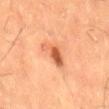Impression:
The lesion was photographed on a routine skin check and not biopsied; there is no pathology result.
Acquisition and patient details:
An algorithmic analysis of the crop reported a mean CIELAB color near L≈54 a*≈27 b*≈36, about 12 CIELAB-L* units darker than the surrounding skin, and a normalized lesion–skin contrast near 8. The analysis additionally found a lesion-detection confidence of about 100/100. The subject is a male aged around 60. This is a cross-polarized tile. Measured at roughly 4 mm in maximum diameter. A 15 mm close-up tile from a total-body photography series done for melanoma screening.